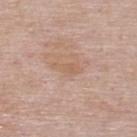Q: Was a biopsy performed?
A: no biopsy performed (imaged during a skin exam)
Q: What is the imaging modality?
A: total-body-photography crop, ~15 mm field of view
Q: Lesion location?
A: the upper back
Q: Automated lesion metrics?
A: border irregularity of about 4.5 on a 0–10 scale, a within-lesion color-variation index near 2/10, and peripheral color asymmetry of about 0.5; a classifier nevus-likeness of about 0/100 and lesion-presence confidence of about 100/100
Q: Lesion size?
A: ≈3 mm
Q: What are the patient's age and sex?
A: male, aged 63–67